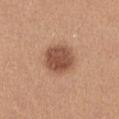This lesion was catalogued during total-body skin photography and was not selected for biopsy.
An algorithmic analysis of the crop reported a footprint of about 12 mm², an eccentricity of roughly 0.45, and a shape-asymmetry score of about 0.1 (0 = symmetric). The analysis additionally found a border-irregularity rating of about 1/10, internal color variation of about 3.5 on a 0–10 scale, and a peripheral color-asymmetry measure near 1. The software also gave a lesion-detection confidence of about 100/100.
From the chest.
This is a white-light tile.
Measured at roughly 4 mm in maximum diameter.
A close-up tile cropped from a whole-body skin photograph, about 15 mm across.
A female patient in their mid- to late 20s.